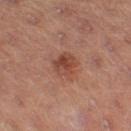No biopsy was performed on this lesion — it was imaged during a full skin examination and was not determined to be concerning. A male subject, aged 53 to 57. A roughly 15 mm field-of-view crop from a total-body skin photograph. The lesion is located on the leg.